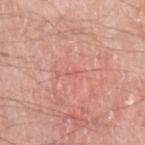  biopsy_status: not biopsied; imaged during a skin examination
  site: left upper arm
  patient:
    sex: male
    age_approx: 60
  image:
    source: total-body photography crop
    field_of_view_mm: 15